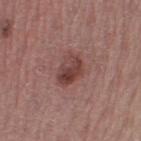{"biopsy_status": "not biopsied; imaged during a skin examination", "patient": {"sex": "female", "age_approx": 55}, "lesion_size": {"long_diameter_mm_approx": 3.5}, "lighting": "white-light", "image": {"source": "total-body photography crop", "field_of_view_mm": 15}, "site": "left thigh"}